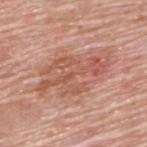Imaged during a routine full-body skin examination; the lesion was not biopsied and no histopathology is available.
The lesion is located on the upper back.
Imaged with white-light lighting.
A roughly 15 mm field-of-view crop from a total-body skin photograph.
Longest diameter approximately 8 mm.
A male patient, aged 78–82.